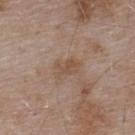Recorded during total-body skin imaging; not selected for excision or biopsy.
A roughly 15 mm field-of-view crop from a total-body skin photograph.
From the upper back.
This is a white-light tile.
A male subject aged approximately 55.